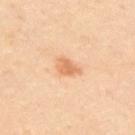This lesion was catalogued during total-body skin photography and was not selected for biopsy.
This is a cross-polarized tile.
A roughly 15 mm field-of-view crop from a total-body skin photograph.
A male patient in their mid-40s.
Automated tile analysis of the lesion measured an average lesion color of about L≈67 a*≈24 b*≈38 (CIELAB), about 10 CIELAB-L* units darker than the surrounding skin, and a normalized lesion–skin contrast near 7. And it measured border irregularity of about 2.5 on a 0–10 scale.
Located on the upper back.
The recorded lesion diameter is about 3 mm.